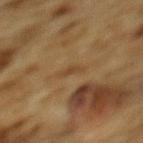biopsy status — catalogued during a skin exam; not biopsied | patient — male, aged approximately 85 or older | tile lighting — cross-polarized | size — ~2.5 mm (longest diameter) | acquisition — 15 mm crop, total-body photography | image-analysis metrics — a border-irregularity rating of about 4/10, a color-variation rating of about 0/10, and radial color variation of about 0; a detector confidence of about 85 out of 100 that the crop contains a lesion | body site — the mid back.